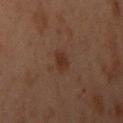Imaged during a routine full-body skin examination; the lesion was not biopsied and no histopathology is available.